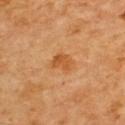Clinical impression:
Part of a total-body skin-imaging series; this lesion was reviewed on a skin check and was not flagged for biopsy.
Image and clinical context:
A 15 mm close-up extracted from a 3D total-body photography capture. The lesion's longest dimension is about 2.5 mm. Located on the upper back. The patient is a male about 60 years old. The tile uses cross-polarized illumination.